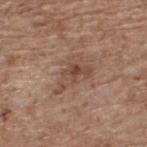Notes:
* notes — total-body-photography surveillance lesion; no biopsy
* lesion size — about 5 mm
* subject — male, aged approximately 70
* lighting — white-light
* image — 15 mm crop, total-body photography
* anatomic site — the upper back